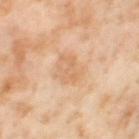  biopsy_status: not biopsied; imaged during a skin examination
  lesion_size:
    long_diameter_mm_approx: 5.0
  automated_metrics:
    border_irregularity_0_10: 3.0
    color_variation_0_10: 3.5
    peripheral_color_asymmetry: 1.0
    nevus_likeness_0_100: 0
    lesion_detection_confidence_0_100: 100
  image:
    source: total-body photography crop
    field_of_view_mm: 15
  patient:
    sex: female
    age_approx: 55
  lighting: cross-polarized
  site: leg